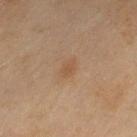illumination: cross-polarized; image: ~15 mm crop, total-body skin-cancer survey; subject: female, aged 58–62; location: the left thigh; size: about 2.5 mm.